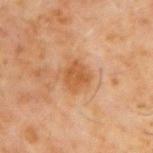Impression: Captured during whole-body skin photography for melanoma surveillance; the lesion was not biopsied. Clinical summary: This is a cross-polarized tile. A lesion tile, about 15 mm wide, cut from a 3D total-body photograph. Approximately 3 mm at its widest. A male subject, aged 58–62. The lesion is located on the upper back.